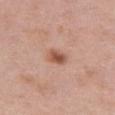biopsy_status: not biopsied; imaged during a skin examination
automated_metrics:
  cielab_L: 54
  cielab_a: 23
  cielab_b: 30
  vs_skin_darker_L: 12.0
  vs_skin_contrast_norm: 8.5
  border_irregularity_0_10: 1.0
  color_variation_0_10: 4.5
  nevus_likeness_0_100: 90
  lesion_detection_confidence_0_100: 100
site: abdomen
patient:
  sex: female
  age_approx: 40
image:
  source: total-body photography crop
  field_of_view_mm: 15